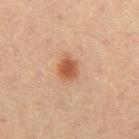• workup: catalogued during a skin exam; not biopsied
• automated metrics: a border-irregularity rating of about 2.5/10, internal color variation of about 6 on a 0–10 scale, and a peripheral color-asymmetry measure near 1.5; an automated nevus-likeness rating near 100 out of 100 and a detector confidence of about 100 out of 100 that the crop contains a lesion
• diameter: ≈4 mm
• acquisition: ~15 mm tile from a whole-body skin photo
• lighting: cross-polarized illumination
• subject: female, aged approximately 20
• body site: the right thigh